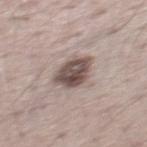notes: catalogued during a skin exam; not biopsied | patient: male, aged 68–72 | illumination: white-light | site: the mid back | acquisition: 15 mm crop, total-body photography.